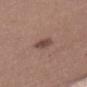notes — no biopsy performed (imaged during a skin exam) | image source — total-body-photography crop, ~15 mm field of view | size — ~3.5 mm (longest diameter) | illumination — white-light | patient — female, aged around 45 | anatomic site — the mid back.